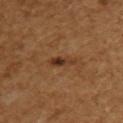Part of a total-body skin-imaging series; this lesion was reviewed on a skin check and was not flagged for biopsy.
On the upper back.
A female subject aged 53–57.
About 3 mm across.
Imaged with cross-polarized lighting.
A 15 mm close-up extracted from a 3D total-body photography capture.
Automated image analysis of the tile measured a lesion area of about 3.5 mm², an outline eccentricity of about 0.9 (0 = round, 1 = elongated), and two-axis asymmetry of about 0.3. And it measured a lesion color around L≈36 a*≈21 b*≈32 in CIELAB, a lesion–skin lightness drop of about 10, and a lesion-to-skin contrast of about 8.5 (normalized; higher = more distinct). And it measured a nevus-likeness score of about 45/100 and a lesion-detection confidence of about 100/100.